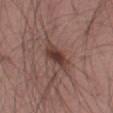Clinical impression: This lesion was catalogued during total-body skin photography and was not selected for biopsy. Clinical summary: On the left thigh. A lesion tile, about 15 mm wide, cut from a 3D total-body photograph. A male subject in their mid-50s. About 4 mm across.